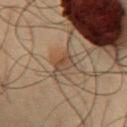Case summary:
– biopsy status — catalogued during a skin exam; not biopsied
– automated metrics — a lesion color around L≈33 a*≈13 b*≈23 in CIELAB, about 6 CIELAB-L* units darker than the surrounding skin, and a normalized border contrast of about 6.5; a border-irregularity rating of about 2.5/10 and peripheral color asymmetry of about 0.5; a nevus-likeness score of about 20/100 and a lesion-detection confidence of about 90/100
– body site — the chest
– image — ~15 mm crop, total-body skin-cancer survey
– illumination — cross-polarized
– size — ≈2.5 mm
– subject — male, aged 48–52